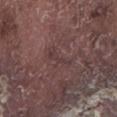Part of a total-body skin-imaging series; this lesion was reviewed on a skin check and was not flagged for biopsy. A 15 mm close-up tile from a total-body photography series done for melanoma screening. The patient is a male aged 73 to 77. The lesion is located on the left lower leg.